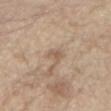  image:
    source: total-body photography crop
    field_of_view_mm: 15
  patient:
    sex: male
    age_approx: 70
  automated_metrics:
    area_mm2_approx: 3.5
    eccentricity: 0.8
    shape_asymmetry: 0.4
    cielab_L: 58
    cielab_a: 15
    cielab_b: 29
    vs_skin_darker_L: 8.0
    vs_skin_contrast_norm: 6.0
    nevus_likeness_0_100: 0
    lesion_detection_confidence_0_100: 95
  lighting: white-light
  lesion_size:
    long_diameter_mm_approx: 3.0
  site: front of the torso